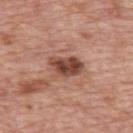Findings:
– patient — male, about 70 years old
– anatomic site — the upper back
– imaging modality — ~15 mm crop, total-body skin-cancer survey